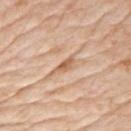workup — catalogued during a skin exam; not biopsied
tile lighting — white-light illumination
acquisition — 15 mm crop, total-body photography
size — about 3 mm
site — the arm
image-analysis metrics — a footprint of about 3.5 mm², a shape eccentricity near 0.9, and a symmetry-axis asymmetry near 0.45; an average lesion color of about L≈62 a*≈20 b*≈34 (CIELAB), roughly 11 lightness units darker than nearby skin, and a normalized lesion–skin contrast near 7; a within-lesion color-variation index near 2.5/10 and a peripheral color-asymmetry measure near 1
patient — male, in their mid- to late 70s